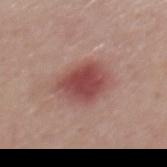Q: Was this lesion biopsied?
A: catalogued during a skin exam; not biopsied
Q: What is the imaging modality?
A: 15 mm crop, total-body photography
Q: How large is the lesion?
A: about 5 mm
Q: Illumination type?
A: white-light illumination
Q: Lesion location?
A: the mid back
Q: Who is the patient?
A: male, in their mid-50s